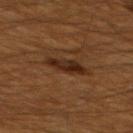Q: Is there a histopathology result?
A: catalogued during a skin exam; not biopsied
Q: Patient demographics?
A: male, aged approximately 60
Q: What is the imaging modality?
A: ~15 mm crop, total-body skin-cancer survey
Q: Illumination type?
A: cross-polarized
Q: Lesion location?
A: the back
Q: Automated lesion metrics?
A: a lesion area of about 6.5 mm², an eccentricity of roughly 0.9, and a shape-asymmetry score of about 0.2 (0 = symmetric); an average lesion color of about L≈24 a*≈18 b*≈25 (CIELAB), a lesion–skin lightness drop of about 9, and a normalized border contrast of about 10.5